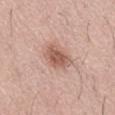Case summary:
* workup · catalogued during a skin exam; not biopsied
* size · about 3.5 mm
* TBP lesion metrics · a border-irregularity index near 2/10, a color-variation rating of about 3/10, and peripheral color asymmetry of about 1
* patient · male, aged around 55
* acquisition · ~15 mm crop, total-body skin-cancer survey
* lighting · white-light illumination